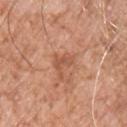biopsy status = catalogued during a skin exam; not biopsied | lighting = white-light | subject = male, aged approximately 55 | lesion size = about 3 mm | image = total-body-photography crop, ~15 mm field of view | body site = the chest.